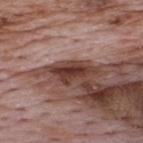Clinical impression: Captured during whole-body skin photography for melanoma surveillance; the lesion was not biopsied. Acquisition and patient details: A 15 mm close-up extracted from a 3D total-body photography capture. A male patient, aged around 70. The lesion is located on the back.